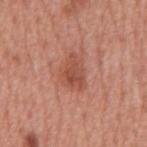biopsy status: no biopsy performed (imaged during a skin exam) | diameter: ≈4 mm | body site: the mid back | illumination: white-light illumination | image source: ~15 mm tile from a whole-body skin photo | patient: male, aged 63 to 67 | image-analysis metrics: a shape-asymmetry score of about 0.3 (0 = symmetric); roughly 9 lightness units darker than nearby skin and a normalized border contrast of about 6.5; a border-irregularity index near 3/10, a color-variation rating of about 3.5/10, and peripheral color asymmetry of about 1; a classifier nevus-likeness of about 75/100 and lesion-presence confidence of about 100/100.